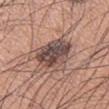follow-up: total-body-photography surveillance lesion; no biopsy
automated metrics: a mean CIELAB color near L≈47 a*≈17 b*≈21, about 14 CIELAB-L* units darker than the surrounding skin, and a normalized lesion–skin contrast near 10.5; border irregularity of about 3 on a 0–10 scale, a within-lesion color-variation index near 7/10, and peripheral color asymmetry of about 2
subject: male, approximately 35 years of age
anatomic site: the right upper arm
diameter: ≈5 mm
acquisition: total-body-photography crop, ~15 mm field of view